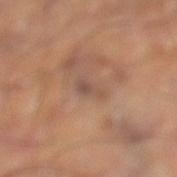Captured during whole-body skin photography for melanoma surveillance; the lesion was not biopsied. A roughly 15 mm field-of-view crop from a total-body skin photograph. From the left thigh.